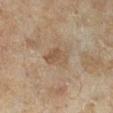The lesion was tiled from a total-body skin photograph and was not biopsied. Cropped from a total-body skin-imaging series; the visible field is about 15 mm. Automated tile analysis of the lesion measured an average lesion color of about L≈47 a*≈14 b*≈29 (CIELAB), a lesion–skin lightness drop of about 7, and a normalized lesion–skin contrast near 6. The analysis additionally found a classifier nevus-likeness of about 0/100 and a lesion-detection confidence of about 100/100. This is a cross-polarized tile. Measured at roughly 3.5 mm in maximum diameter. The patient is a female in their 60s. Located on the right lower leg.